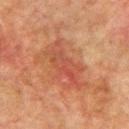follow-up — total-body-photography surveillance lesion; no biopsy
automated lesion analysis — a lesion color around L≈43 a*≈25 b*≈30 in CIELAB, roughly 7 lightness units darker than nearby skin, and a normalized border contrast of about 5.5
imaging modality — 15 mm crop, total-body photography
size — ≈6 mm
site — the right upper arm
patient — male, aged approximately 75
illumination — cross-polarized illumination